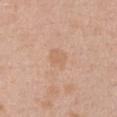Recorded during total-body skin imaging; not selected for excision or biopsy.
The total-body-photography lesion software estimated a footprint of about 4.5 mm², an outline eccentricity of about 0.6 (0 = round, 1 = elongated), and a shape-asymmetry score of about 0.25 (0 = symmetric). And it measured border irregularity of about 2.5 on a 0–10 scale, a within-lesion color-variation index near 1.5/10, and radial color variation of about 0.5.
A roughly 15 mm field-of-view crop from a total-body skin photograph.
A female patient, aged 23 to 27.
On the chest.
Imaged with white-light lighting.
Measured at roughly 2.5 mm in maximum diameter.